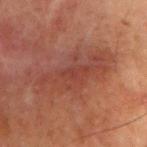follow-up: catalogued during a skin exam; not biopsied | tile lighting: cross-polarized | location: the arm | subject: male, roughly 60 years of age | imaging modality: 15 mm crop, total-body photography.